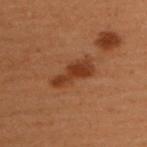Q: Was a biopsy performed?
A: no biopsy performed (imaged during a skin exam)
Q: Lesion location?
A: the upper back
Q: What lighting was used for the tile?
A: cross-polarized
Q: How was this image acquired?
A: ~15 mm crop, total-body skin-cancer survey
Q: Patient demographics?
A: female, approximately 50 years of age